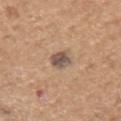Q: Was this lesion biopsied?
A: total-body-photography surveillance lesion; no biopsy
Q: Lesion size?
A: about 3 mm
Q: Who is the patient?
A: male, in their mid-60s
Q: How was the tile lit?
A: white-light
Q: Where on the body is the lesion?
A: the arm
Q: What kind of image is this?
A: 15 mm crop, total-body photography